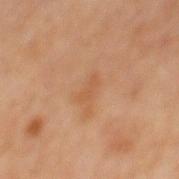No biopsy was performed on this lesion — it was imaged during a full skin examination and was not determined to be concerning. Located on the mid back. About 2.5 mm across. A region of skin cropped from a whole-body photographic capture, roughly 15 mm wide. Imaged with cross-polarized lighting. A male patient about 60 years old.